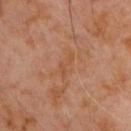The lesion was photographed on a routine skin check and not biopsied; there is no pathology result.
Located on the chest.
A 15 mm crop from a total-body photograph taken for skin-cancer surveillance.
The subject is a male in their 60s.